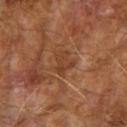The total-body-photography lesion software estimated a normalized lesion–skin contrast near 5.5. And it measured an automated nevus-likeness rating near 0 out of 100 and a lesion-detection confidence of about 90/100. From the right upper arm. The recorded lesion diameter is about 3.5 mm. A male patient, about 65 years old. Imaged with cross-polarized lighting. This image is a 15 mm lesion crop taken from a total-body photograph.